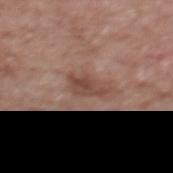Imaged during a routine full-body skin examination; the lesion was not biopsied and no histopathology is available.
A region of skin cropped from a whole-body photographic capture, roughly 15 mm wide.
The patient is a male aged approximately 70.
The lesion is on the mid back.
The lesion's longest dimension is about 4 mm.
Imaged with white-light lighting.